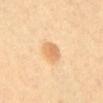workup = no biopsy performed (imaged during a skin exam) | automated lesion analysis = roughly 10 lightness units darker than nearby skin and a normalized lesion–skin contrast near 6.5; a nevus-likeness score of about 90/100 and a lesion-detection confidence of about 100/100 | image = total-body-photography crop, ~15 mm field of view | size = ~3 mm (longest diameter) | tile lighting = cross-polarized illumination | subject = aged 58 to 62 | location = the abdomen.